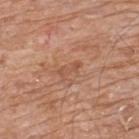Recorded during total-body skin imaging; not selected for excision or biopsy.
About 3 mm across.
From the upper back.
Imaged with white-light lighting.
The patient is a male aged approximately 80.
Cropped from a whole-body photographic skin survey; the tile spans about 15 mm.
The total-body-photography lesion software estimated roughly 7 lightness units darker than nearby skin and a normalized border contrast of about 5. The software also gave a classifier nevus-likeness of about 0/100 and a lesion-detection confidence of about 100/100.